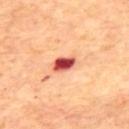Q: Was this lesion biopsied?
A: total-body-photography surveillance lesion; no biopsy
Q: Patient demographics?
A: male, approximately 70 years of age
Q: How large is the lesion?
A: ~3 mm (longest diameter)
Q: How was this image acquired?
A: total-body-photography crop, ~15 mm field of view
Q: How was the tile lit?
A: cross-polarized illumination
Q: Where on the body is the lesion?
A: the upper back